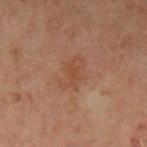Recorded during total-body skin imaging; not selected for excision or biopsy. This is a cross-polarized tile. From the arm. The subject is a male aged 43 to 47. This image is a 15 mm lesion crop taken from a total-body photograph. An algorithmic analysis of the crop reported a footprint of about 8.5 mm² and two-axis asymmetry of about 0.25. The software also gave radial color variation of about 0.5. The software also gave a nevus-likeness score of about 0/100.